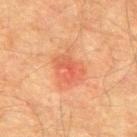biopsy status — no biopsy performed (imaged during a skin exam) | lesion size — ≈3.5 mm | automated lesion analysis — a lesion area of about 6 mm², an outline eccentricity of about 0.75 (0 = round, 1 = elongated), and a shape-asymmetry score of about 0.35 (0 = symmetric); about 8 CIELAB-L* units darker than the surrounding skin and a lesion-to-skin contrast of about 5.5 (normalized; higher = more distinct); a border-irregularity index near 4.5/10, a within-lesion color-variation index near 4.5/10, and radial color variation of about 1.5 | subject — male, in their mid- to late 70s | site — the upper back | imaging modality — ~15 mm tile from a whole-body skin photo.